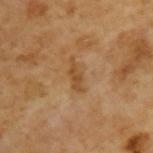The lesion was tiled from a total-body skin photograph and was not biopsied.
A male subject approximately 65 years of age.
Measured at roughly 3.5 mm in maximum diameter.
A close-up tile cropped from a whole-body skin photograph, about 15 mm across.
This is a cross-polarized tile.